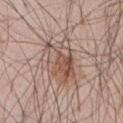Captured during whole-body skin photography for melanoma surveillance; the lesion was not biopsied. The tile uses white-light illumination. On the abdomen. The recorded lesion diameter is about 6 mm. A 15 mm close-up extracted from a 3D total-body photography capture. Automated image analysis of the tile measured a footprint of about 15 mm². The analysis additionally found a border-irregularity rating of about 4.5/10 and a color-variation rating of about 8/10. The software also gave a nevus-likeness score of about 65/100 and lesion-presence confidence of about 100/100. The patient is a male aged 53 to 57.